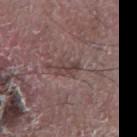Q: Was this lesion biopsied?
A: imaged on a skin check; not biopsied
Q: What did automated image analysis measure?
A: roughly 8 lightness units darker than nearby skin and a lesion-to-skin contrast of about 7 (normalized; higher = more distinct); a border-irregularity rating of about 3.5/10 and a within-lesion color-variation index near 0/10
Q: Who is the patient?
A: male, in their mid-60s
Q: Where on the body is the lesion?
A: the arm
Q: What is the imaging modality?
A: 15 mm crop, total-body photography
Q: Illumination type?
A: white-light